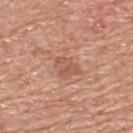Case summary:
– follow-up: catalogued during a skin exam; not biopsied
– image source: ~15 mm tile from a whole-body skin photo
– size: ≈3 mm
– tile lighting: white-light illumination
– subject: male, roughly 60 years of age
– body site: the upper back